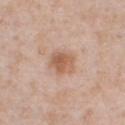| field | value |
|---|---|
| workup | no biopsy performed (imaged during a skin exam) |
| subject | male, about 50 years old |
| illumination | white-light illumination |
| site | the chest |
| image | 15 mm crop, total-body photography |
| size | ≈3 mm |